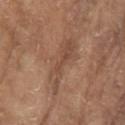Assessment: The lesion was photographed on a routine skin check and not biopsied; there is no pathology result. Clinical summary: Approximately 6.5 mm at its widest. Located on the left upper arm. A male patient approximately 80 years of age. This is a white-light tile. A close-up tile cropped from a whole-body skin photograph, about 15 mm across. Automated image analysis of the tile measured an eccentricity of roughly 0.95 and a symmetry-axis asymmetry near 0.4. And it measured a classifier nevus-likeness of about 0/100 and a lesion-detection confidence of about 70/100.